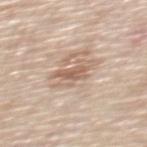Case summary:
• notes · catalogued during a skin exam; not biopsied
• subject · male, aged 53–57
• automated lesion analysis · a border-irregularity rating of about 6.5/10, a color-variation rating of about 4.5/10, and radial color variation of about 1.5; an automated nevus-likeness rating near 0 out of 100 and a lesion-detection confidence of about 75/100
• image · ~15 mm crop, total-body skin-cancer survey
• anatomic site · the upper back
• diameter · ≈4.5 mm
• lighting · white-light